A female patient aged around 65. A lesion tile, about 15 mm wide, cut from a 3D total-body photograph. On the mid back.
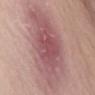The tile uses white-light illumination.
The biopsy diagnosis was a solar lentigo — a benign lesion.